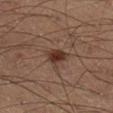{
  "biopsy_status": "not biopsied; imaged during a skin examination",
  "image": {
    "source": "total-body photography crop",
    "field_of_view_mm": 15
  },
  "lighting": "cross-polarized",
  "lesion_size": {
    "long_diameter_mm_approx": 2.5
  },
  "site": "right lower leg",
  "automated_metrics": {
    "color_variation_0_10": 2.0,
    "peripheral_color_asymmetry": 0.5
  },
  "patient": {
    "sex": "male",
    "age_approx": 60
  }
}